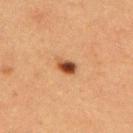This lesion was catalogued during total-body skin photography and was not selected for biopsy.
A female patient, aged 38 to 42.
The lesion is on the back.
A 15 mm close-up tile from a total-body photography series done for melanoma screening.
This is a cross-polarized tile.
About 2.5 mm across.
An algorithmic analysis of the crop reported a lesion area of about 3.5 mm² and an eccentricity of roughly 0.8. The analysis additionally found an average lesion color of about L≈40 a*≈23 b*≈32 (CIELAB) and about 15 CIELAB-L* units darker than the surrounding skin. It also reported a classifier nevus-likeness of about 100/100 and a detector confidence of about 100 out of 100 that the crop contains a lesion.